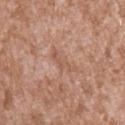No biopsy was performed on this lesion — it was imaged during a full skin examination and was not determined to be concerning. On the arm. About 3.5 mm across. A lesion tile, about 15 mm wide, cut from a 3D total-body photograph. The lesion-visualizer software estimated a border-irregularity rating of about 6.5/10 and internal color variation of about 0 on a 0–10 scale. It also reported a nevus-likeness score of about 0/100 and a detector confidence of about 95 out of 100 that the crop contains a lesion. The subject is a male in their mid-40s. Imaged with white-light lighting.